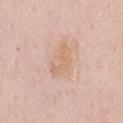Captured during whole-body skin photography for melanoma surveillance; the lesion was not biopsied. An algorithmic analysis of the crop reported a classifier nevus-likeness of about 0/100. Cropped from a total-body skin-imaging series; the visible field is about 15 mm. A male subject, aged 48 to 52. The lesion is on the chest.